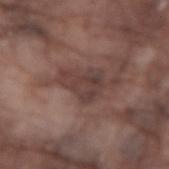{
  "biopsy_status": "not biopsied; imaged during a skin examination",
  "image": {
    "source": "total-body photography crop",
    "field_of_view_mm": 15
  },
  "patient": {
    "sex": "male",
    "age_approx": 75
  },
  "lesion_size": {
    "long_diameter_mm_approx": 4.0
  },
  "site": "right forearm",
  "lighting": "white-light"
}